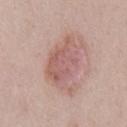| key | value |
|---|---|
| illumination | white-light |
| site | the chest |
| subject | male, aged 38 to 42 |
| diameter | ≈6.5 mm |
| imaging modality | total-body-photography crop, ~15 mm field of view |